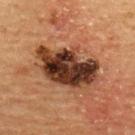Cropped from a whole-body photographic skin survey; the tile spans about 15 mm. The tile uses cross-polarized illumination. A female patient, aged around 70. From the upper back. The recorded lesion diameter is about 7 mm.